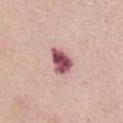A female subject roughly 65 years of age. Approximately 3.5 mm at its widest. A 15 mm crop from a total-body photograph taken for skin-cancer surveillance. Located on the abdomen.